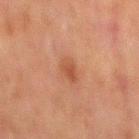Clinical impression: The lesion was photographed on a routine skin check and not biopsied; there is no pathology result. Background: A male patient, aged 58 to 62. The tile uses cross-polarized illumination. From the mid back. A 15 mm crop from a total-body photograph taken for skin-cancer surveillance.